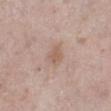Clinical impression:
This lesion was catalogued during total-body skin photography and was not selected for biopsy.
Image and clinical context:
Longest diameter approximately 2.5 mm. The total-body-photography lesion software estimated an eccentricity of roughly 0.65 and a shape-asymmetry score of about 0.3 (0 = symmetric). And it measured a lesion-detection confidence of about 100/100. This is a white-light tile. The subject is a male aged around 60. The lesion is on the left lower leg. A 15 mm close-up extracted from a 3D total-body photography capture.